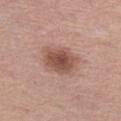Assessment: Imaged during a routine full-body skin examination; the lesion was not biopsied and no histopathology is available. Background: The recorded lesion diameter is about 4.5 mm. The tile uses white-light illumination. The subject is a female roughly 65 years of age. The lesion is on the left thigh. A 15 mm close-up tile from a total-body photography series done for melanoma screening.